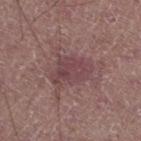Impression:
The lesion was photographed on a routine skin check and not biopsied; there is no pathology result.
Image and clinical context:
The lesion is on the left lower leg. A lesion tile, about 15 mm wide, cut from a 3D total-body photograph. Measured at roughly 4.5 mm in maximum diameter. The tile uses white-light illumination. A male patient, roughly 60 years of age.